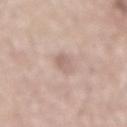No biopsy was performed on this lesion — it was imaged during a full skin examination and was not determined to be concerning.
A close-up tile cropped from a whole-body skin photograph, about 15 mm across.
From the mid back.
The patient is a male aged 68–72.